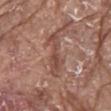workup=imaged on a skin check; not biopsied
image-analysis metrics=a lesion color around L≈48 a*≈22 b*≈26 in CIELAB, a lesion–skin lightness drop of about 9, and a normalized border contrast of about 6.5; a border-irregularity rating of about 5.5/10, internal color variation of about 3 on a 0–10 scale, and a peripheral color-asymmetry measure near 1; a classifier nevus-likeness of about 0/100
imaging modality=~15 mm crop, total-body skin-cancer survey
patient=male, roughly 80 years of age
tile lighting=white-light illumination
anatomic site=the mid back
lesion diameter=~5.5 mm (longest diameter)